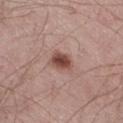This is a white-light tile.
Located on the right thigh.
A roughly 15 mm field-of-view crop from a total-body skin photograph.
The patient is a male aged 63–67.
Automated tile analysis of the lesion measured an eccentricity of roughly 0.35 and two-axis asymmetry of about 0.2. The software also gave a border-irregularity rating of about 2/10, a color-variation rating of about 4/10, and radial color variation of about 1. And it measured an automated nevus-likeness rating near 95 out of 100 and a lesion-detection confidence of about 100/100.
Measured at roughly 2.5 mm in maximum diameter.